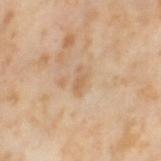Imaged during a routine full-body skin examination; the lesion was not biopsied and no histopathology is available. On the left thigh. A female subject about 55 years old. Approximately 3 mm at its widest. A 15 mm crop from a total-body photograph taken for skin-cancer surveillance. The tile uses cross-polarized illumination.